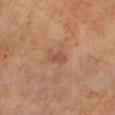Imaged during a routine full-body skin examination; the lesion was not biopsied and no histopathology is available.
The tile uses cross-polarized illumination.
This image is a 15 mm lesion crop taken from a total-body photograph.
The total-body-photography lesion software estimated a lesion area of about 3.5 mm² and an eccentricity of roughly 0.85. The software also gave roughly 7 lightness units darker than nearby skin and a lesion-to-skin contrast of about 5.5 (normalized; higher = more distinct). And it measured a border-irregularity index near 4/10, internal color variation of about 1.5 on a 0–10 scale, and a peripheral color-asymmetry measure near 0.5. The analysis additionally found lesion-presence confidence of about 100/100.
A male subject, about 65 years old.
Longest diameter approximately 2.5 mm.
From the left lower leg.